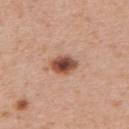Impression: This lesion was catalogued during total-body skin photography and was not selected for biopsy. Background: A female subject aged approximately 30. Located on the upper back. A 15 mm crop from a total-body photograph taken for skin-cancer surveillance.